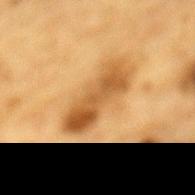workup — no biopsy performed (imaged during a skin exam).